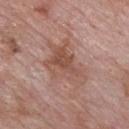The lesion was photographed on a routine skin check and not biopsied; there is no pathology result.
Cropped from a total-body skin-imaging series; the visible field is about 15 mm.
A male subject approximately 60 years of age.
From the front of the torso.
The tile uses white-light illumination.